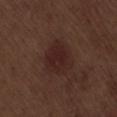follow-up=catalogued during a skin exam; not biopsied | subject=male, aged 68 to 72 | location=the lower back | acquisition=15 mm crop, total-body photography.